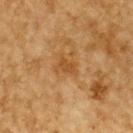– workup — no biopsy performed (imaged during a skin exam)
– lighting — cross-polarized illumination
– image source — total-body-photography crop, ~15 mm field of view
– diameter — ~2.5 mm (longest diameter)
– subject — male, in their mid-80s or older
– body site — the upper back